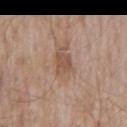Recorded during total-body skin imaging; not selected for excision or biopsy.
The lesion's longest dimension is about 3 mm.
This image is a 15 mm lesion crop taken from a total-body photograph.
Captured under white-light illumination.
A male patient, about 65 years old.
On the mid back.